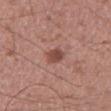{
  "biopsy_status": "not biopsied; imaged during a skin examination",
  "site": "right upper arm",
  "patient": {
    "sex": "male",
    "age_approx": 35
  },
  "image": {
    "source": "total-body photography crop",
    "field_of_view_mm": 15
  }
}